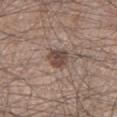Case summary:
* notes — imaged on a skin check; not biopsied
* lesion diameter — about 3 mm
* patient — male, in their mid-40s
* imaging modality — ~15 mm tile from a whole-body skin photo
* site — the left lower leg
* tile lighting — white-light
* automated metrics — a lesion area of about 5.5 mm², a shape eccentricity near 0.5, and a symmetry-axis asymmetry near 0.25; a border-irregularity rating of about 2/10, a color-variation rating of about 3.5/10, and a peripheral color-asymmetry measure near 1